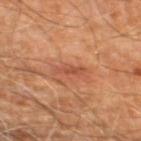Findings:
- workup: no biopsy performed (imaged during a skin exam)
- automated metrics: a lesion area of about 3 mm² and an eccentricity of roughly 0.9; a border-irregularity index near 4/10 and a color-variation rating of about 0/10
- illumination: cross-polarized illumination
- anatomic site: the right leg
- size: about 3 mm
- image source: ~15 mm crop, total-body skin-cancer survey
- subject: male, about 60 years old